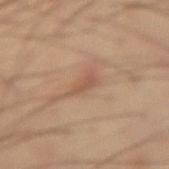Assessment: This lesion was catalogued during total-body skin photography and was not selected for biopsy. Background: This image is a 15 mm lesion crop taken from a total-body photograph. A male subject, aged 53–57. Longest diameter approximately 3 mm. On the left thigh. Captured under cross-polarized illumination.